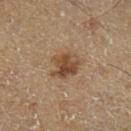Part of a total-body skin-imaging series; this lesion was reviewed on a skin check and was not flagged for biopsy. Automated image analysis of the tile measured an eccentricity of roughly 0.5. It also reported a mean CIELAB color near L≈37 a*≈14 b*≈26 and a lesion–skin lightness drop of about 9. The software also gave a detector confidence of about 100 out of 100 that the crop contains a lesion. A male patient roughly 65 years of age. The lesion is on the left lower leg. Longest diameter approximately 4 mm. A 15 mm crop from a total-body photograph taken for skin-cancer surveillance.